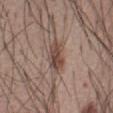Image and clinical context: Cropped from a total-body skin-imaging series; the visible field is about 15 mm. Located on the abdomen. A male subject aged around 55.